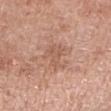<record>
<patient>
  <sex>female</sex>
  <age_approx>60</age_approx>
</patient>
<site>right forearm</site>
<image>
  <source>total-body photography crop</source>
  <field_of_view_mm>15</field_of_view_mm>
</image>
<lighting>white-light</lighting>
</record>